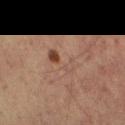The lesion was photographed on a routine skin check and not biopsied; there is no pathology result. A close-up tile cropped from a whole-body skin photograph, about 15 mm across. Located on the left thigh. The tile uses cross-polarized illumination. A male subject, aged 58 to 62. An algorithmic analysis of the crop reported a lesion area of about 6.5 mm², an eccentricity of roughly 0.9, and a shape-asymmetry score of about 0.35 (0 = symmetric). The analysis additionally found a lesion color around L≈39 a*≈16 b*≈23 in CIELAB, roughly 6 lightness units darker than nearby skin, and a normalized lesion–skin contrast near 5. The analysis additionally found a border-irregularity rating of about 5/10, a color-variation rating of about 10/10, and radial color variation of about 3.5. It also reported an automated nevus-likeness rating near 20 out of 100. The recorded lesion diameter is about 4.5 mm.